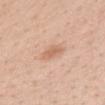workup — catalogued during a skin exam; not biopsied | imaging modality — ~15 mm tile from a whole-body skin photo | lesion size — ≈3.5 mm | TBP lesion metrics — an eccentricity of roughly 0.8 and a shape-asymmetry score of about 0.25 (0 = symmetric); a lesion color around L≈65 a*≈21 b*≈33 in CIELAB, roughly 9 lightness units darker than nearby skin, and a lesion-to-skin contrast of about 6 (normalized; higher = more distinct) | subject — female, aged 33–37 | illumination — white-light illumination | site — the mid back.